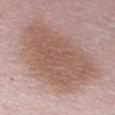image=~15 mm crop, total-body skin-cancer survey
diameter=≈11 mm
tile lighting=white-light illumination
body site=the right upper arm
patient=male, roughly 65 years of age